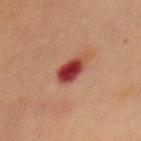  patient:
    sex: female
    age_approx: 55
  site: chest
  automated_metrics:
    cielab_L: 32
    cielab_a: 29
    cielab_b: 23
    vs_skin_darker_L: 15.0
    nevus_likeness_0_100: 0
    lesion_detection_confidence_0_100: 100
  lighting: cross-polarized
  image:
    source: total-body photography crop
    field_of_view_mm: 15
  lesion_size:
    long_diameter_mm_approx: 3.0